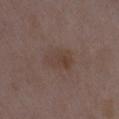| field | value |
|---|---|
| biopsy status | catalogued during a skin exam; not biopsied |
| imaging modality | ~15 mm crop, total-body skin-cancer survey |
| lesion diameter | about 3.5 mm |
| image-analysis metrics | an automated nevus-likeness rating near 0 out of 100 and a lesion-detection confidence of about 100/100 |
| subject | female, in their mid-30s |
| location | the right thigh |
| lighting | white-light |